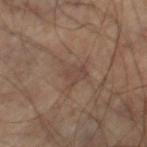– biopsy status: imaged on a skin check; not biopsied
– diameter: about 3.5 mm
– location: the right thigh
– subject: male, aged 58–62
– automated lesion analysis: a mean CIELAB color near L≈42 a*≈16 b*≈23 and about 6 CIELAB-L* units darker than the surrounding skin; a border-irregularity rating of about 5.5/10, a within-lesion color-variation index near 2/10, and peripheral color asymmetry of about 1; a classifier nevus-likeness of about 0/100 and a detector confidence of about 95 out of 100 that the crop contains a lesion
– image source: 15 mm crop, total-body photography
– illumination: cross-polarized